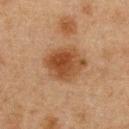Clinical impression: The lesion was tiled from a total-body skin photograph and was not biopsied. Acquisition and patient details: The lesion is located on the abdomen. Cropped from a total-body skin-imaging series; the visible field is about 15 mm. A male patient, in their mid-70s.